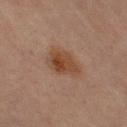workup = imaged on a skin check; not biopsied | site = the leg | image source = ~15 mm crop, total-body skin-cancer survey | tile lighting = cross-polarized | lesion diameter = ≈5 mm | subject = female, roughly 70 years of age.